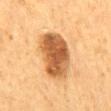This lesion was catalogued during total-body skin photography and was not selected for biopsy.
This image is a 15 mm lesion crop taken from a total-body photograph.
On the mid back.
Captured under cross-polarized illumination.
A male subject aged 58 to 62.